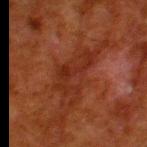biopsy_status: not biopsied; imaged during a skin examination
site: left thigh
image:
  source: total-body photography crop
  field_of_view_mm: 15
patient:
  sex: male
  age_approx: 80